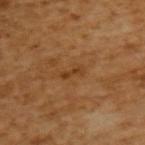No biopsy was performed on this lesion — it was imaged during a full skin examination and was not determined to be concerning. The patient is a male aged approximately 65. Automated image analysis of the tile measured an outline eccentricity of about 0.95 (0 = round, 1 = elongated). Captured under cross-polarized illumination. A 15 mm crop from a total-body photograph taken for skin-cancer surveillance.